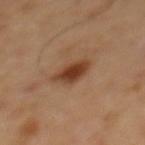| key | value |
|---|---|
| follow-up | no biopsy performed (imaged during a skin exam) |
| patient | male, in their mid- to late 60s |
| automated lesion analysis | a mean CIELAB color near L≈40 a*≈22 b*≈32 and a lesion-to-skin contrast of about 10 (normalized; higher = more distinct); a classifier nevus-likeness of about 100/100 |
| lighting | cross-polarized |
| acquisition | total-body-photography crop, ~15 mm field of view |
| size | about 3.5 mm |
| body site | the mid back |